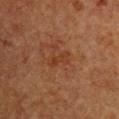Findings:
* follow-up — imaged on a skin check; not biopsied
* acquisition — ~15 mm tile from a whole-body skin photo
* automated lesion analysis — an area of roughly 2.5 mm² and an eccentricity of roughly 0.95; a classifier nevus-likeness of about 0/100 and a detector confidence of about 100 out of 100 that the crop contains a lesion
* location — the chest
* illumination — cross-polarized
* lesion size — ≈3 mm
* subject — female, aged around 70